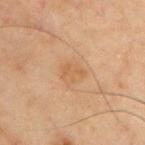follow-up = catalogued during a skin exam; not biopsied | image-analysis metrics = a classifier nevus-likeness of about 0/100 and a detector confidence of about 100 out of 100 that the crop contains a lesion | patient = male, in their mid-50s | anatomic site = the chest | image source = 15 mm crop, total-body photography.